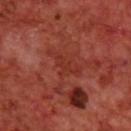biopsy_status: not biopsied; imaged during a skin examination
image:
  source: total-body photography crop
  field_of_view_mm: 15
lesion_size:
  long_diameter_mm_approx: 3.0
lighting: cross-polarized
site: back
patient:
  sex: male
  age_approx: 70